Approximately 6 mm at its widest; An algorithmic analysis of the crop reported a normalized border contrast of about 7. It also reported a border-irregularity index near 9/10 and internal color variation of about 5.5 on a 0–10 scale. And it measured a nevus-likeness score of about 0/100 and a detector confidence of about 95 out of 100 that the crop contains a lesion; a female subject, aged 53 to 57; the tile uses white-light illumination; a lesion tile, about 15 mm wide, cut from a 3D total-body photograph; located on the right lower leg.
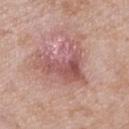Notes:
* pathology · a dermatofibroma — a benign skin lesion